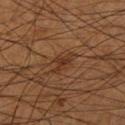Captured during whole-body skin photography for melanoma surveillance; the lesion was not biopsied.
The lesion-visualizer software estimated an average lesion color of about L≈31 a*≈18 b*≈28 (CIELAB), a lesion–skin lightness drop of about 7, and a normalized lesion–skin contrast near 6.5. The analysis additionally found a border-irregularity rating of about 5/10 and peripheral color asymmetry of about 0.5.
Longest diameter approximately 3.5 mm.
The patient is a male approximately 55 years of age.
Located on the right lower leg.
A 15 mm close-up extracted from a 3D total-body photography capture.
This is a cross-polarized tile.